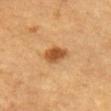The lesion was tiled from a total-body skin photograph and was not biopsied. A male patient roughly 85 years of age or older. A lesion tile, about 15 mm wide, cut from a 3D total-body photograph. From the left upper arm.